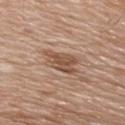<case>
<biopsy_status>not biopsied; imaged during a skin examination</biopsy_status>
<lesion_size>
  <long_diameter_mm_approx>5.0</long_diameter_mm_approx>
</lesion_size>
<patient>
  <sex>male</sex>
  <age_approx>80</age_approx>
</patient>
<lighting>white-light</lighting>
<site>chest</site>
<image>
  <source>total-body photography crop</source>
  <field_of_view_mm>15</field_of_view_mm>
</image>
<automated_metrics>
  <area_mm2_approx>9.0</area_mm2_approx>
  <eccentricity>0.9</eccentricity>
  <cielab_L>52</cielab_L>
  <cielab_a>19</cielab_a>
  <cielab_b>30</cielab_b>
  <vs_skin_darker_L>10.0</vs_skin_darker_L>
  <border_irregularity_0_10>3.5</border_irregularity_0_10>
  <color_variation_0_10>3.5</color_variation_0_10>
  <peripheral_color_asymmetry>1.0</peripheral_color_asymmetry>
  <nevus_likeness_0_100>0</nevus_likeness_0_100>
  <lesion_detection_confidence_0_100>100</lesion_detection_confidence_0_100>
</automated_metrics>
</case>